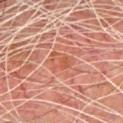{"biopsy_status": "not biopsied; imaged during a skin examination", "site": "chest", "image": {"source": "total-body photography crop", "field_of_view_mm": 15}, "patient": {"sex": "male", "age_approx": 80}, "lesion_size": {"long_diameter_mm_approx": 3.0}}